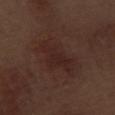The lesion was photographed on a routine skin check and not biopsied; there is no pathology result.
Located on the left thigh.
Cropped from a whole-body photographic skin survey; the tile spans about 15 mm.
A male subject roughly 70 years of age.
Automated image analysis of the tile measured a lesion area of about 14 mm² and an outline eccentricity of about 0.85 (0 = round, 1 = elongated). The analysis additionally found a color-variation rating of about 2/10 and radial color variation of about 0.5. And it measured a nevus-likeness score of about 0/100 and lesion-presence confidence of about 100/100.
The recorded lesion diameter is about 6 mm.